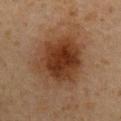Recorded during total-body skin imaging; not selected for excision or biopsy.
Cropped from a whole-body photographic skin survey; the tile spans about 15 mm.
An algorithmic analysis of the crop reported a footprint of about 28 mm², a shape eccentricity near 0.45, and two-axis asymmetry of about 0.25. The analysis additionally found a lesion–skin lightness drop of about 10. The software also gave a nevus-likeness score of about 100/100.
This is a cross-polarized tile.
The lesion is located on the front of the torso.
A male patient approximately 60 years of age.